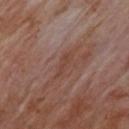{"biopsy_status": "not biopsied; imaged during a skin examination", "image": {"source": "total-body photography crop", "field_of_view_mm": 15}, "site": "upper back", "patient": {"sex": "male", "age_approx": 70}, "lighting": "cross-polarized", "lesion_size": {"long_diameter_mm_approx": 3.0}}